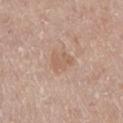Part of a total-body skin-imaging series; this lesion was reviewed on a skin check and was not flagged for biopsy. A female subject aged 58 to 62. Cropped from a whole-body photographic skin survey; the tile spans about 15 mm. The lesion is on the left lower leg. Automated tile analysis of the lesion measured a footprint of about 4.5 mm² and an outline eccentricity of about 0.8 (0 = round, 1 = elongated). It also reported border irregularity of about 3.5 on a 0–10 scale. The analysis additionally found a detector confidence of about 100 out of 100 that the crop contains a lesion. Approximately 2.5 mm at its widest. This is a white-light tile.